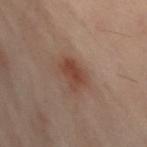Findings:
- workup — imaged on a skin check; not biopsied
- image source — ~15 mm crop, total-body skin-cancer survey
- site — the left leg
- patient — female, aged 58–62
- lighting — cross-polarized